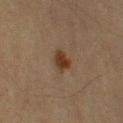biopsy status: imaged on a skin check; not biopsied
lesion diameter: ~3 mm (longest diameter)
illumination: cross-polarized illumination
body site: the right upper arm
automated metrics: an automated nevus-likeness rating near 95 out of 100 and lesion-presence confidence of about 100/100
patient: male, aged 63 to 67
image: ~15 mm crop, total-body skin-cancer survey